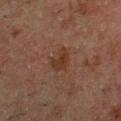Recorded during total-body skin imaging; not selected for excision or biopsy.